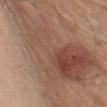Clinical impression: This lesion was catalogued during total-body skin photography and was not selected for biopsy. Background: Located on the arm. A male patient, aged 48 to 52. This is a white-light tile. A region of skin cropped from a whole-body photographic capture, roughly 15 mm wide. Approximately 18.5 mm at its widest. Automated tile analysis of the lesion measured a lesion area of about 85 mm², a shape eccentricity near 0.8, and a shape-asymmetry score of about 0.55 (0 = symmetric). And it measured border irregularity of about 9 on a 0–10 scale, a color-variation rating of about 8/10, and a peripheral color-asymmetry measure near 2.5.